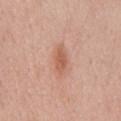  site: mid back
  patient:
    sex: male
    age_approx: 70
  automated_metrics:
    area_mm2_approx: 6.0
    eccentricity: 0.85
    shape_asymmetry: 0.2
    cielab_L: 59
    cielab_a: 24
    cielab_b: 31
    vs_skin_darker_L: 9.0
    vs_skin_contrast_norm: 6.5
    border_irregularity_0_10: 2.5
    color_variation_0_10: 2.0
    peripheral_color_asymmetry: 0.5
  image:
    source: total-body photography crop
    field_of_view_mm: 15